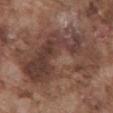Impression: The lesion was photographed on a routine skin check and not biopsied; there is no pathology result. Background: The subject is a male roughly 75 years of age. An algorithmic analysis of the crop reported an area of roughly 55 mm², an eccentricity of roughly 0.85, and two-axis asymmetry of about 0.45. The software also gave a mean CIELAB color near L≈40 a*≈18 b*≈23 and a normalized lesion–skin contrast near 8. It also reported border irregularity of about 8.5 on a 0–10 scale. The recorded lesion diameter is about 11.5 mm. A close-up tile cropped from a whole-body skin photograph, about 15 mm across. Imaged with white-light lighting. The lesion is located on the chest.